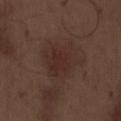A male subject, about 70 years old. The lesion is on the abdomen. A roughly 15 mm field-of-view crop from a total-body skin photograph.